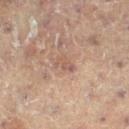{
  "biopsy_status": "not biopsied; imaged during a skin examination",
  "image": {
    "source": "total-body photography crop",
    "field_of_view_mm": 15
  },
  "site": "right leg",
  "lighting": "cross-polarized",
  "lesion_size": {
    "long_diameter_mm_approx": 2.5
  },
  "patient": {
    "sex": "female",
    "age_approx": 80
  }
}